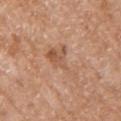Q: Was a biopsy performed?
A: catalogued during a skin exam; not biopsied
Q: What lighting was used for the tile?
A: white-light illumination
Q: What did automated image analysis measure?
A: an average lesion color of about L≈56 a*≈21 b*≈33 (CIELAB), roughly 7 lightness units darker than nearby skin, and a lesion-to-skin contrast of about 5.5 (normalized; higher = more distinct); a border-irregularity index near 6.5/10 and a color-variation rating of about 4/10
Q: How was this image acquired?
A: 15 mm crop, total-body photography
Q: What are the patient's age and sex?
A: female, aged approximately 75
Q: How large is the lesion?
A: ~6 mm (longest diameter)
Q: Where on the body is the lesion?
A: the right upper arm